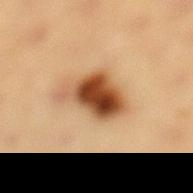The lesion was tiled from a total-body skin photograph and was not biopsied.